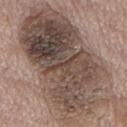workup = total-body-photography surveillance lesion; no biopsy
site = the back
lesion size = ~15 mm (longest diameter)
TBP lesion metrics = a footprint of about 95 mm² and a shape eccentricity near 0.85; a nevus-likeness score of about 0/100 and a detector confidence of about 95 out of 100 that the crop contains a lesion
lighting = white-light illumination
acquisition = ~15 mm tile from a whole-body skin photo
patient = male, aged 68 to 72